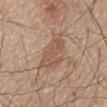Q: Was this lesion biopsied?
A: total-body-photography surveillance lesion; no biopsy
Q: Automated lesion metrics?
A: a border-irregularity rating of about 3.5/10, a within-lesion color-variation index near 3/10, and a peripheral color-asymmetry measure near 1
Q: Where on the body is the lesion?
A: the mid back
Q: What kind of image is this?
A: ~15 mm tile from a whole-body skin photo
Q: Who is the patient?
A: male, in their mid- to late 60s